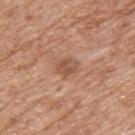Case summary:
* tile lighting — white-light
* size — ≈2.5 mm
* automated metrics — a footprint of about 4.5 mm², an outline eccentricity of about 0.6 (0 = round, 1 = elongated), and a shape-asymmetry score of about 0.2 (0 = symmetric); a lesion color around L≈52 a*≈22 b*≈30 in CIELAB, a lesion–skin lightness drop of about 9, and a normalized border contrast of about 6.5; a classifier nevus-likeness of about 10/100 and lesion-presence confidence of about 100/100
* subject — male, about 60 years old
* anatomic site — the upper back
* acquisition — ~15 mm tile from a whole-body skin photo